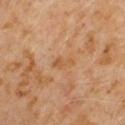follow-up: total-body-photography surveillance lesion; no biopsy
diameter: ~2.5 mm (longest diameter)
tile lighting: cross-polarized illumination
subject: male, in their 60s
location: the right upper arm
image-analysis metrics: a lesion area of about 2.5 mm², an outline eccentricity of about 0.9 (0 = round, 1 = elongated), and a symmetry-axis asymmetry near 0.45; a classifier nevus-likeness of about 0/100
image: 15 mm crop, total-body photography